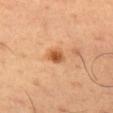Recorded during total-body skin imaging; not selected for excision or biopsy.
A male patient in their 50s.
Captured under cross-polarized illumination.
Longest diameter approximately 2.5 mm.
The total-body-photography lesion software estimated roughly 13 lightness units darker than nearby skin and a normalized lesion–skin contrast near 8.5.
A lesion tile, about 15 mm wide, cut from a 3D total-body photograph.
From the left thigh.